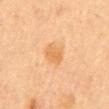Assessment:
This lesion was catalogued during total-body skin photography and was not selected for biopsy.
Acquisition and patient details:
A female patient, approximately 60 years of age. Cropped from a total-body skin-imaging series; the visible field is about 15 mm. The lesion is located on the mid back. Longest diameter approximately 2.5 mm.